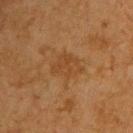workup — imaged on a skin check; not biopsied | subject — male, in their mid- to late 70s | TBP lesion metrics — a footprint of about 8 mm², an eccentricity of roughly 0.75, and two-axis asymmetry of about 0.3; an average lesion color of about L≈35 a*≈17 b*≈31 (CIELAB) and a lesion–skin lightness drop of about 5; a nevus-likeness score of about 0/100 | image — 15 mm crop, total-body photography | site — the upper back | illumination — cross-polarized | diameter — ≈4 mm.